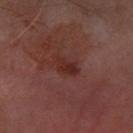No biopsy was performed on this lesion — it was imaged during a full skin examination and was not determined to be concerning. About 3 mm across. Located on the left forearm. This is a cross-polarized tile. A male subject, aged 63–67. A 15 mm close-up extracted from a 3D total-body photography capture.